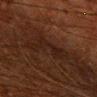Recorded during total-body skin imaging; not selected for excision or biopsy. A male patient roughly 60 years of age. Captured under cross-polarized illumination. A lesion tile, about 15 mm wide, cut from a 3D total-body photograph. Longest diameter approximately 5 mm. From the right upper arm.